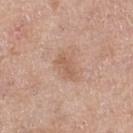{"image": {"source": "total-body photography crop", "field_of_view_mm": 15}, "site": "leg", "patient": {"sex": "female", "age_approx": 65}}